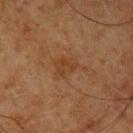workup = imaged on a skin check; not biopsied
imaging modality = ~15 mm crop, total-body skin-cancer survey
patient = male, about 65 years old
size = about 4 mm
lighting = cross-polarized
location = the left upper arm